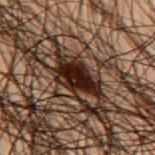biopsy status: total-body-photography surveillance lesion; no biopsy
acquisition: ~15 mm tile from a whole-body skin photo
body site: the back
patient: male, aged approximately 50
lighting: cross-polarized illumination
image-analysis metrics: a lesion area of about 9.5 mm², a shape eccentricity near 0.85, and a shape-asymmetry score of about 0.35 (0 = symmetric); a color-variation rating of about 4/10 and peripheral color asymmetry of about 1.5
diameter: ≈4.5 mm